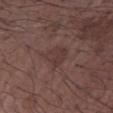<case>
<biopsy_status>not biopsied; imaged during a skin examination</biopsy_status>
<image>
  <source>total-body photography crop</source>
  <field_of_view_mm>15</field_of_view_mm>
</image>
<lesion_size>
  <long_diameter_mm_approx>3.0</long_diameter_mm_approx>
</lesion_size>
<automated_metrics>
  <vs_skin_darker_L>5.0</vs_skin_darker_L>
  <vs_skin_contrast_norm>5.0</vs_skin_contrast_norm>
  <nevus_likeness_0_100>0</nevus_likeness_0_100>
  <lesion_detection_confidence_0_100>100</lesion_detection_confidence_0_100>
</automated_metrics>
<lighting>white-light</lighting>
<patient>
  <sex>male</sex>
  <age_approx>70</age_approx>
</patient>
<site>right upper arm</site>
</case>